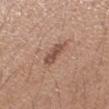The lesion was photographed on a routine skin check and not biopsied; there is no pathology result. An algorithmic analysis of the crop reported an average lesion color of about L≈51 a*≈20 b*≈27 (CIELAB), a lesion–skin lightness drop of about 10, and a lesion-to-skin contrast of about 7.5 (normalized; higher = more distinct). It also reported a border-irregularity rating of about 2.5/10. And it measured a classifier nevus-likeness of about 45/100 and lesion-presence confidence of about 100/100. A female subject, aged around 25. Captured under white-light illumination. On the right forearm. A close-up tile cropped from a whole-body skin photograph, about 15 mm across.